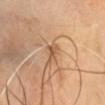- workup: total-body-photography surveillance lesion; no biopsy
- subject: male, approximately 60 years of age
- image: ~15 mm tile from a whole-body skin photo
- anatomic site: the head or neck